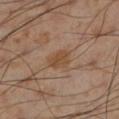workup: no biopsy performed (imaged during a skin exam)
acquisition: ~15 mm tile from a whole-body skin photo
patient: male, aged 53–57
lesion size: ≈3 mm
lighting: cross-polarized
location: the left lower leg
automated metrics: a footprint of about 5.5 mm², an eccentricity of roughly 0.7, and two-axis asymmetry of about 0.2; a lesion color around L≈47 a*≈18 b*≈32 in CIELAB and a lesion-to-skin contrast of about 7 (normalized; higher = more distinct)